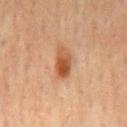Imaged during a routine full-body skin examination; the lesion was not biopsied and no histopathology is available. This is a cross-polarized tile. A 15 mm close-up extracted from a 3D total-body photography capture. The subject is a male aged 68–72. Approximately 4 mm at its widest. The lesion is on the front of the torso.